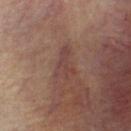biopsy status: catalogued during a skin exam; not biopsied | tile lighting: cross-polarized | automated lesion analysis: a footprint of about 6.5 mm² and two-axis asymmetry of about 0.55; a border-irregularity rating of about 6/10, a within-lesion color-variation index near 2/10, and radial color variation of about 0.5 | subject: female, roughly 70 years of age | image: ~15 mm tile from a whole-body skin photo | lesion size: about 4 mm | location: the head or neck.